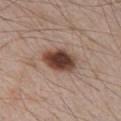Part of a total-body skin-imaging series; this lesion was reviewed on a skin check and was not flagged for biopsy. A male patient, aged approximately 65. Imaged with white-light lighting. Automated tile analysis of the lesion measured border irregularity of about 2 on a 0–10 scale, internal color variation of about 6.5 on a 0–10 scale, and radial color variation of about 1.5. A 15 mm crop from a total-body photograph taken for skin-cancer surveillance. The recorded lesion diameter is about 5 mm. Located on the chest.